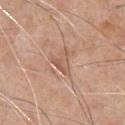Q: Was this lesion biopsied?
A: total-body-photography surveillance lesion; no biopsy
Q: What lighting was used for the tile?
A: white-light illumination
Q: Where on the body is the lesion?
A: the front of the torso
Q: Lesion size?
A: ≈3 mm
Q: What is the imaging modality?
A: total-body-photography crop, ~15 mm field of view
Q: What are the patient's age and sex?
A: male, aged 63 to 67